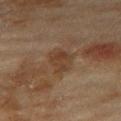Clinical summary: This image is a 15 mm lesion crop taken from a total-body photograph. Approximately 3.5 mm at its widest. Located on the right thigh. A female patient in their 60s. Imaged with cross-polarized lighting. The lesion-visualizer software estimated an eccentricity of roughly 0.6 and two-axis asymmetry of about 0.25. It also reported a lesion color around L≈36 a*≈16 b*≈27 in CIELAB, roughly 6 lightness units darker than nearby skin, and a normalized border contrast of about 6. And it measured a border-irregularity index near 2.5/10, a within-lesion color-variation index near 2.5/10, and peripheral color asymmetry of about 0.5.